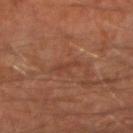Q: Was this lesion biopsied?
A: total-body-photography surveillance lesion; no biopsy
Q: Who is the patient?
A: male, aged around 65
Q: What is the anatomic site?
A: the right forearm
Q: How was this image acquired?
A: 15 mm crop, total-body photography